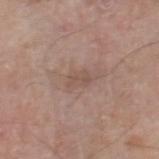Context:
The total-body-photography lesion software estimated a footprint of about 3.5 mm². The analysis additionally found an average lesion color of about L≈52 a*≈17 b*≈24 (CIELAB), about 6 CIELAB-L* units darker than the surrounding skin, and a lesion-to-skin contrast of about 5 (normalized; higher = more distinct). Captured under white-light illumination. A region of skin cropped from a whole-body photographic capture, roughly 15 mm wide. A male subject aged 78–82. From the right thigh.